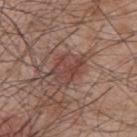The lesion was photographed on a routine skin check and not biopsied; there is no pathology result.
Longest diameter approximately 4.5 mm.
The subject is a male aged 48 to 52.
A close-up tile cropped from a whole-body skin photograph, about 15 mm across.
The lesion is on the upper back.